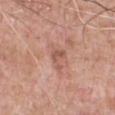| feature | finding |
|---|---|
| follow-up | catalogued during a skin exam; not biopsied |
| subject | male, aged approximately 60 |
| site | the chest |
| acquisition | ~15 mm crop, total-body skin-cancer survey |
| image-analysis metrics | a footprint of about 3.5 mm², an eccentricity of roughly 0.75, and a symmetry-axis asymmetry near 0.5; border irregularity of about 5.5 on a 0–10 scale, a within-lesion color-variation index near 2.5/10, and a peripheral color-asymmetry measure near 1; lesion-presence confidence of about 100/100 |
| lesion size | ~3 mm (longest diameter) |